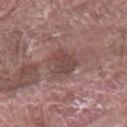<tbp_lesion>
  <automated_metrics>
    <border_irregularity_0_10>4.0</border_irregularity_0_10>
    <color_variation_0_10>1.5</color_variation_0_10>
    <peripheral_color_asymmetry>0.5</peripheral_color_asymmetry>
  </automated_metrics>
  <site>arm</site>
  <patient>
    <sex>male</sex>
    <age_approx>75</age_approx>
  </patient>
  <lighting>white-light</lighting>
  <image>
    <source>total-body photography crop</source>
    <field_of_view_mm>15</field_of_view_mm>
  </image>
  <lesion_size>
    <long_diameter_mm_approx>3.5</long_diameter_mm_approx>
  </lesion_size>
</tbp_lesion>